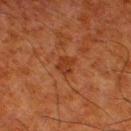Assessment:
This lesion was catalogued during total-body skin photography and was not selected for biopsy.
Background:
A male subject in their 80s. Cropped from a whole-body photographic skin survey; the tile spans about 15 mm. The tile uses cross-polarized illumination. The recorded lesion diameter is about 2.5 mm. An algorithmic analysis of the crop reported an eccentricity of roughly 0.5. The analysis additionally found an automated nevus-likeness rating near 0 out of 100. The lesion is located on the leg.